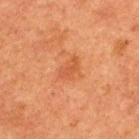Case summary:
• follow-up: imaged on a skin check; not biopsied
• image source: ~15 mm crop, total-body skin-cancer survey
• site: the upper back
• subject: male, aged approximately 50